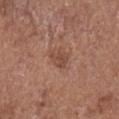Captured during whole-body skin photography for melanoma surveillance; the lesion was not biopsied. A 15 mm close-up extracted from a 3D total-body photography capture. The lesion is on the right lower leg. Automated image analysis of the tile measured a shape eccentricity near 0.65. It also reported an average lesion color of about L≈47 a*≈22 b*≈27 (CIELAB) and a normalized border contrast of about 6. The software also gave border irregularity of about 4 on a 0–10 scale and internal color variation of about 2 on a 0–10 scale. This is a white-light tile. A male patient, approximately 75 years of age.